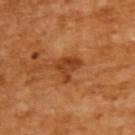Part of a total-body skin-imaging series; this lesion was reviewed on a skin check and was not flagged for biopsy.
On the upper back.
A close-up tile cropped from a whole-body skin photograph, about 15 mm across.
The patient is a female in their mid-50s.